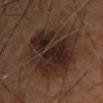About 9.5 mm across.
Captured under cross-polarized illumination.
A male patient aged approximately 60.
This image is a 15 mm lesion crop taken from a total-body photograph.
From the chest.